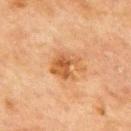workup: no biopsy performed (imaged during a skin exam); image-analysis metrics: a border-irregularity rating of about 4.5/10, internal color variation of about 5 on a 0–10 scale, and peripheral color asymmetry of about 1.5; anatomic site: the mid back; lesion diameter: ~4 mm (longest diameter); acquisition: ~15 mm crop, total-body skin-cancer survey; tile lighting: cross-polarized illumination; subject: male, roughly 70 years of age.